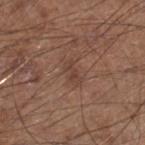Q: What is the lesion's diameter?
A: ~3 mm (longest diameter)
Q: What are the patient's age and sex?
A: male, in their 60s
Q: What did automated image analysis measure?
A: a within-lesion color-variation index near 0/10 and radial color variation of about 0; a lesion-detection confidence of about 100/100
Q: What is the anatomic site?
A: the right lower leg
Q: What is the imaging modality?
A: 15 mm crop, total-body photography